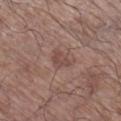Q: Was a biopsy performed?
A: no biopsy performed (imaged during a skin exam)
Q: How was this image acquired?
A: total-body-photography crop, ~15 mm field of view
Q: Lesion location?
A: the right lower leg
Q: Patient demographics?
A: male, aged 63 to 67
Q: Illumination type?
A: white-light
Q: What is the lesion's diameter?
A: ≈3 mm
Q: Automated lesion metrics?
A: a lesion color around L≈47 a*≈19 b*≈23 in CIELAB, a lesion–skin lightness drop of about 7, and a lesion-to-skin contrast of about 5.5 (normalized; higher = more distinct); a within-lesion color-variation index near 1.5/10 and peripheral color asymmetry of about 0.5; a lesion-detection confidence of about 100/100